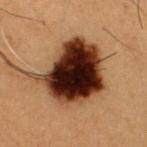Clinical impression:
No biopsy was performed on this lesion — it was imaged during a full skin examination and was not determined to be concerning.
Image and clinical context:
A close-up tile cropped from a whole-body skin photograph, about 15 mm across. Longest diameter approximately 7.5 mm. Automated tile analysis of the lesion measured a lesion area of about 37 mm², an eccentricity of roughly 0.5, and a symmetry-axis asymmetry near 0.25. It also reported a border-irregularity rating of about 3/10, internal color variation of about 10 on a 0–10 scale, and radial color variation of about 3. And it measured a nevus-likeness score of about 25/100 and lesion-presence confidence of about 100/100. The lesion is located on the chest. A male subject aged approximately 50. This is a cross-polarized tile.